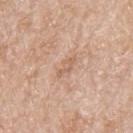Context:
Cropped from a whole-body photographic skin survey; the tile spans about 15 mm. This is a white-light tile. The lesion is on the upper back. The subject is a male aged approximately 80. About 3 mm across. The total-body-photography lesion software estimated a lesion–skin lightness drop of about 7. And it measured a nevus-likeness score of about 0/100.